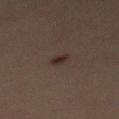The lesion was tiled from a total-body skin photograph and was not biopsied.
Captured under cross-polarized illumination.
On the mid back.
A region of skin cropped from a whole-body photographic capture, roughly 15 mm wide.
A male subject, aged 28 to 32.
About 2 mm across.